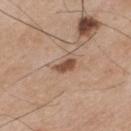Impression:
Captured during whole-body skin photography for melanoma surveillance; the lesion was not biopsied.
Image and clinical context:
Cropped from a whole-body photographic skin survey; the tile spans about 15 mm. Approximately 3 mm at its widest. A male subject about 75 years old. The tile uses white-light illumination. The lesion-visualizer software estimated an average lesion color of about L≈50 a*≈20 b*≈30 (CIELAB) and a normalized border contrast of about 9.5. And it measured border irregularity of about 2.5 on a 0–10 scale, a within-lesion color-variation index near 1.5/10, and radial color variation of about 0.5. It also reported an automated nevus-likeness rating near 85 out of 100. Located on the mid back.